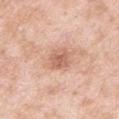biopsy status = total-body-photography surveillance lesion; no biopsy | subject = male, in their mid- to late 50s | location = the arm | lighting = white-light illumination | imaging modality = ~15 mm tile from a whole-body skin photo.